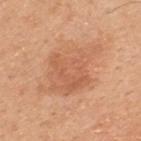This lesion was catalogued during total-body skin photography and was not selected for biopsy. Cropped from a whole-body photographic skin survey; the tile spans about 15 mm. The tile uses white-light illumination. A male patient, aged 48 to 52. From the upper back. The lesion's longest dimension is about 8 mm.